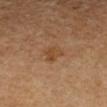follow-up: imaged on a skin check; not biopsied
image-analysis metrics: an outline eccentricity of about 0.6 (0 = round, 1 = elongated) and a shape-asymmetry score of about 0.25 (0 = symmetric); a mean CIELAB color near L≈44 a*≈19 b*≈34 and a lesion–skin lightness drop of about 7
site: the left forearm
lesion size: ≈2.5 mm
patient: female, about 50 years old
imaging modality: ~15 mm tile from a whole-body skin photo